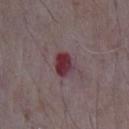Q: Was a biopsy performed?
A: catalogued during a skin exam; not biopsied
Q: Patient demographics?
A: male, approximately 65 years of age
Q: What is the anatomic site?
A: the chest
Q: What kind of image is this?
A: 15 mm crop, total-body photography
Q: How was the tile lit?
A: white-light
Q: What is the lesion's diameter?
A: ≈2.5 mm